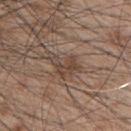Imaged during a routine full-body skin examination; the lesion was not biopsied and no histopathology is available.
Cropped from a whole-body photographic skin survey; the tile spans about 15 mm.
A male subject, in their 50s.
The lesion is on the chest.
An algorithmic analysis of the crop reported a footprint of about 5.5 mm² and a symmetry-axis asymmetry near 0.55. The software also gave a border-irregularity rating of about 7/10, a within-lesion color-variation index near 2.5/10, and a peripheral color-asymmetry measure near 0.5.
Captured under white-light illumination.